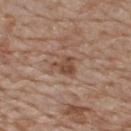| field | value |
|---|---|
| follow-up | imaged on a skin check; not biopsied |
| automated metrics | an area of roughly 5.5 mm², an eccentricity of roughly 0.6, and a shape-asymmetry score of about 0.4 (0 = symmetric); border irregularity of about 4 on a 0–10 scale and internal color variation of about 3.5 on a 0–10 scale; a classifier nevus-likeness of about 15/100 |
| anatomic site | the upper back |
| tile lighting | white-light illumination |
| size | ~3 mm (longest diameter) |
| patient | male, aged 78–82 |
| imaging modality | ~15 mm tile from a whole-body skin photo |